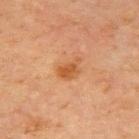  biopsy_status: not biopsied; imaged during a skin examination
  site: left upper arm
  automated_metrics:
    border_irregularity_0_10: 3.5
    color_variation_0_10: 2.0
    peripheral_color_asymmetry: 0.5
    nevus_likeness_0_100: 45
    lesion_detection_confidence_0_100: 100
  image:
    source: total-body photography crop
    field_of_view_mm: 15
  patient:
    sex: male
    age_approx: 65